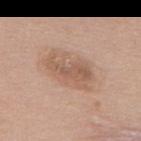Recorded during total-body skin imaging; not selected for excision or biopsy.
Longest diameter approximately 6 mm.
The lesion is located on the upper back.
This image is a 15 mm lesion crop taken from a total-body photograph.
Imaged with white-light lighting.
Automated image analysis of the tile measured a lesion area of about 15 mm², an outline eccentricity of about 0.85 (0 = round, 1 = elongated), and a shape-asymmetry score of about 0.2 (0 = symmetric). It also reported a within-lesion color-variation index near 4/10 and radial color variation of about 1.5.
A female patient about 60 years old.